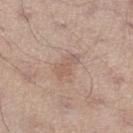{"site": "right lower leg", "patient": {"sex": "male", "age_approx": 35}, "lighting": "white-light", "image": {"source": "total-body photography crop", "field_of_view_mm": 15}, "lesion_size": {"long_diameter_mm_approx": 3.5}}